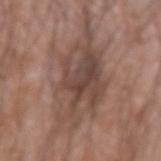Imaged during a routine full-body skin examination; the lesion was not biopsied and no histopathology is available. On the left forearm. Cropped from a whole-body photographic skin survey; the tile spans about 15 mm. The total-body-photography lesion software estimated an area of roughly 34 mm², a shape eccentricity near 0.9, and a shape-asymmetry score of about 0.35 (0 = symmetric). And it measured a lesion color around L≈46 a*≈17 b*≈23 in CIELAB and a lesion–skin lightness drop of about 10. The analysis additionally found border irregularity of about 7.5 on a 0–10 scale, a color-variation rating of about 6/10, and a peripheral color-asymmetry measure near 2. The software also gave a nevus-likeness score of about 0/100 and lesion-presence confidence of about 50/100. The subject is a male in their 60s. The recorded lesion diameter is about 12.5 mm.